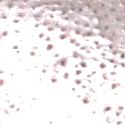Captured during whole-body skin photography for melanoma surveillance; the lesion was not biopsied. The patient is a male about 50 years old. A lesion tile, about 15 mm wide, cut from a 3D total-body photograph. From the head or neck. Approximately 1.5 mm at its widest. The tile uses white-light illumination.